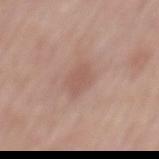The lesion was photographed on a routine skin check and not biopsied; there is no pathology result. Captured under white-light illumination. The total-body-photography lesion software estimated a within-lesion color-variation index near 1.5/10 and a peripheral color-asymmetry measure near 0.5. About 2.5 mm across. From the mid back. A male subject roughly 60 years of age. Cropped from a total-body skin-imaging series; the visible field is about 15 mm.